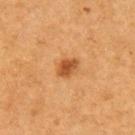follow-up=no biopsy performed (imaged during a skin exam)
anatomic site=the arm
lighting=cross-polarized
image=~15 mm crop, total-body skin-cancer survey
patient=male, about 55 years old